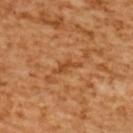Case summary:
• follow-up: no biopsy performed (imaged during a skin exam)
• patient: female, about 55 years old
• lesion size: about 3.5 mm
• body site: the back
• lighting: cross-polarized
• image source: ~15 mm crop, total-body skin-cancer survey